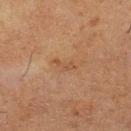{
  "biopsy_status": "not biopsied; imaged during a skin examination",
  "image": {
    "source": "total-body photography crop",
    "field_of_view_mm": 15
  },
  "patient": {
    "sex": "female",
    "age_approx": 50
  },
  "site": "left lower leg"
}